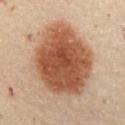Captured during whole-body skin photography for melanoma surveillance; the lesion was not biopsied.
The lesion's longest dimension is about 9.5 mm.
This is a cross-polarized tile.
On the abdomen.
A 15 mm close-up extracted from a 3D total-body photography capture.
Automated image analysis of the tile measured a mean CIELAB color near L≈53 a*≈22 b*≈33, a lesion–skin lightness drop of about 17, and a lesion-to-skin contrast of about 11 (normalized; higher = more distinct). The analysis additionally found internal color variation of about 5.5 on a 0–10 scale and peripheral color asymmetry of about 1.5.
The patient is a female approximately 45 years of age.